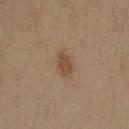The lesion was photographed on a routine skin check and not biopsied; there is no pathology result.
The tile uses cross-polarized illumination.
The lesion's longest dimension is about 3 mm.
A lesion tile, about 15 mm wide, cut from a 3D total-body photograph.
Located on the left arm.
The patient is a male in their 50s.